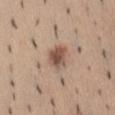| feature | finding |
|---|---|
| follow-up | no biopsy performed (imaged during a skin exam) |